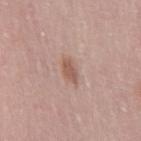Impression:
Part of a total-body skin-imaging series; this lesion was reviewed on a skin check and was not flagged for biopsy.
Background:
A female patient aged around 30. On the leg. A 15 mm close-up extracted from a 3D total-body photography capture. This is a white-light tile.